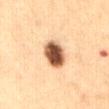{"biopsy_status": "not biopsied; imaged during a skin examination", "patient": {"sex": "female", "age_approx": 30}, "lighting": "cross-polarized", "site": "abdomen", "image": {"source": "total-body photography crop", "field_of_view_mm": 15}, "automated_metrics": {"vs_skin_contrast_norm": 14.5, "border_irregularity_0_10": 1.5, "color_variation_0_10": 7.0}}